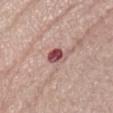Clinical summary: The patient is a female aged 63 to 67. About 3 mm across. The tile uses white-light illumination. The lesion is located on the abdomen. Cropped from a whole-body photographic skin survey; the tile spans about 15 mm.